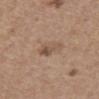Assessment:
The lesion was photographed on a routine skin check and not biopsied; there is no pathology result.
Clinical summary:
A female patient, about 65 years old. An algorithmic analysis of the crop reported a footprint of about 5 mm², a shape eccentricity near 0.8, and a shape-asymmetry score of about 0.4 (0 = symmetric). And it measured a lesion color around L≈52 a*≈16 b*≈28 in CIELAB, roughly 8 lightness units darker than nearby skin, and a lesion-to-skin contrast of about 6 (normalized; higher = more distinct). And it measured a border-irregularity index near 4/10, a within-lesion color-variation index near 6/10, and a peripheral color-asymmetry measure near 2.5. This is a white-light tile. A lesion tile, about 15 mm wide, cut from a 3D total-body photograph. About 3 mm across. On the abdomen.